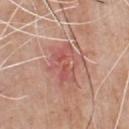follow-up: imaged on a skin check; not biopsied | subject: male, aged approximately 80 | automated lesion analysis: an area of roughly 10 mm², a shape eccentricity near 0.8, and a shape-asymmetry score of about 0.35 (0 = symmetric); a lesion color around L≈55 a*≈28 b*≈26 in CIELAB and a normalized lesion–skin contrast near 5.5; a border-irregularity index near 5.5/10, a color-variation rating of about 5.5/10, and a peripheral color-asymmetry measure near 2 | anatomic site: the chest | image source: ~15 mm crop, total-body skin-cancer survey | illumination: white-light.